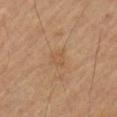The lesion was tiled from a total-body skin photograph and was not biopsied. The tile uses cross-polarized illumination. A lesion tile, about 15 mm wide, cut from a 3D total-body photograph. About 2.5 mm across. A male subject in their mid- to late 50s. The lesion is on the left upper arm.